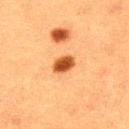biopsy_status: not biopsied; imaged during a skin examination
image:
  source: total-body photography crop
  field_of_view_mm: 15
automated_metrics:
  area_mm2_approx: 4.5
  shape_asymmetry: 0.2
  nevus_likeness_0_100: 100
  lesion_detection_confidence_0_100: 100
lighting: cross-polarized
patient:
  sex: male
  age_approx: 40
lesion_size:
  long_diameter_mm_approx: 2.5
site: upper back